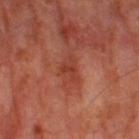Q: Is there a histopathology result?
A: no biopsy performed (imaged during a skin exam)
Q: Illumination type?
A: cross-polarized
Q: What are the patient's age and sex?
A: male, aged around 70
Q: What kind of image is this?
A: ~15 mm crop, total-body skin-cancer survey
Q: Automated lesion metrics?
A: an area of roughly 3.5 mm² and a shape-asymmetry score of about 0.5 (0 = symmetric); a mean CIELAB color near L≈40 a*≈30 b*≈31
Q: Lesion location?
A: the right thigh